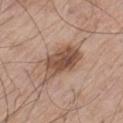Impression:
Imaged during a routine full-body skin examination; the lesion was not biopsied and no histopathology is available.
Acquisition and patient details:
Cropped from a whole-body photographic skin survey; the tile spans about 15 mm. The tile uses white-light illumination. From the left thigh. The recorded lesion diameter is about 7 mm. The total-body-photography lesion software estimated a lesion area of about 15 mm², a shape eccentricity near 0.9, and a shape-asymmetry score of about 0.3 (0 = symmetric). It also reported an average lesion color of about L≈51 a*≈19 b*≈28 (CIELAB). The analysis additionally found border irregularity of about 3.5 on a 0–10 scale, a within-lesion color-variation index near 6/10, and radial color variation of about 2.5. The software also gave a classifier nevus-likeness of about 25/100 and lesion-presence confidence of about 100/100. The patient is a male aged approximately 80.